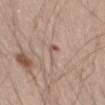| feature | finding |
|---|---|
| lighting | white-light |
| image-analysis metrics | a lesion area of about 3.5 mm² and two-axis asymmetry of about 0.65; a mean CIELAB color near L≈58 a*≈17 b*≈24, about 7 CIELAB-L* units darker than the surrounding skin, and a lesion-to-skin contrast of about 5 (normalized; higher = more distinct); a border-irregularity index near 7.5/10, a color-variation rating of about 5/10, and radial color variation of about 1.5 |
| size | about 3.5 mm |
| site | the left thigh |
| subject | male, roughly 60 years of age |
| acquisition | 15 mm crop, total-body photography |